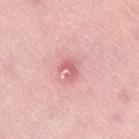biopsy_status: not biopsied; imaged during a skin examination
patient:
  sex: female
  age_approx: 60
lighting: white-light
image:
  source: total-body photography crop
  field_of_view_mm: 15
automated_metrics:
  cielab_L: 63
  cielab_a: 31
  cielab_b: 21
  vs_skin_contrast_norm: 6.0
  color_variation_0_10: 1.0
  peripheral_color_asymmetry: 0.5
site: lower back